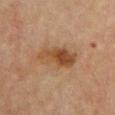Assessment:
Captured during whole-body skin photography for melanoma surveillance; the lesion was not biopsied.
Clinical summary:
A lesion tile, about 15 mm wide, cut from a 3D total-body photograph. The lesion is on the chest. The subject is a female aged 68–72.